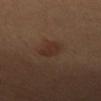No biopsy was performed on this lesion — it was imaged during a full skin examination and was not determined to be concerning.
From the arm.
Captured under cross-polarized illumination.
This image is a 15 mm lesion crop taken from a total-body photograph.
An algorithmic analysis of the crop reported an area of roughly 5.5 mm² and a symmetry-axis asymmetry near 0.3. It also reported a within-lesion color-variation index near 1.5/10.
A female subject roughly 40 years of age.